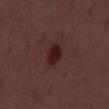Impression:
The lesion was tiled from a total-body skin photograph and was not biopsied.
Acquisition and patient details:
The subject is a male in their 50s. This image is a 15 mm lesion crop taken from a total-body photograph. Imaged with white-light lighting.